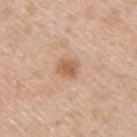{"biopsy_status": "not biopsied; imaged during a skin examination", "patient": {"sex": "male", "age_approx": 50}, "lesion_size": {"long_diameter_mm_approx": 2.5}, "automated_metrics": {"cielab_L": 60, "cielab_a": 21, "cielab_b": 34, "vs_skin_darker_L": 10.0, "vs_skin_contrast_norm": 7.0, "border_irregularity_0_10": 2.0, "color_variation_0_10": 2.5, "peripheral_color_asymmetry": 1.0, "nevus_likeness_0_100": 65, "lesion_detection_confidence_0_100": 100}, "image": {"source": "total-body photography crop", "field_of_view_mm": 15}, "site": "upper back", "lighting": "white-light"}